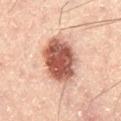automated_metrics:
  area_mm2_approx: 21.0
  shape_asymmetry: 0.15
  border_irregularity_0_10: 2.0
  peripheral_color_asymmetry: 2.0
  lesion_detection_confidence_0_100: 100
patient:
  sex: male
  age_approx: 50
lighting: cross-polarized
site: leg
image:
  source: total-body photography crop
  field_of_view_mm: 15